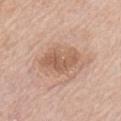– biopsy status — total-body-photography surveillance lesion; no biopsy
– tile lighting — white-light
– acquisition — ~15 mm crop, total-body skin-cancer survey
– body site — the left upper arm
– patient — male, about 75 years old